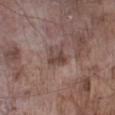The tile uses white-light illumination. The lesion is on the left lower leg. The recorded lesion diameter is about 2.5 mm. A male patient, aged around 75. A 15 mm close-up extracted from a 3D total-body photography capture.